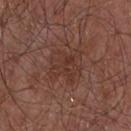follow-up=imaged on a skin check; not biopsied | lesion diameter=about 4 mm | subject=male, in their mid- to late 50s | body site=the chest | image source=~15 mm tile from a whole-body skin photo.